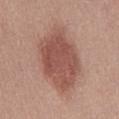follow-up = catalogued during a skin exam; not biopsied | location = the abdomen | image source = total-body-photography crop, ~15 mm field of view | tile lighting = white-light illumination | lesion diameter = ~8 mm (longest diameter) | patient = male, aged 43 to 47 | TBP lesion metrics = a peripheral color-asymmetry measure near 1; a lesion-detection confidence of about 100/100.